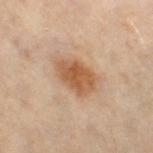Q: Was this lesion biopsied?
A: no biopsy performed (imaged during a skin exam)
Q: Who is the patient?
A: female, aged 48–52
Q: What is the anatomic site?
A: the right thigh
Q: What did automated image analysis measure?
A: a within-lesion color-variation index near 3.5/10 and radial color variation of about 1
Q: How was this image acquired?
A: total-body-photography crop, ~15 mm field of view
Q: What lighting was used for the tile?
A: cross-polarized
Q: Lesion size?
A: ~5 mm (longest diameter)